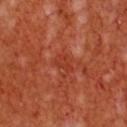Q: Was this lesion biopsied?
A: no biopsy performed (imaged during a skin exam)
Q: What kind of image is this?
A: 15 mm crop, total-body photography
Q: What is the lesion's diameter?
A: about 2.5 mm
Q: Who is the patient?
A: male, about 55 years old
Q: What did automated image analysis measure?
A: a shape eccentricity near 0.75 and a symmetry-axis asymmetry near 0.35; a mean CIELAB color near L≈42 a*≈36 b*≈37, a lesion–skin lightness drop of about 6, and a lesion-to-skin contrast of about 5.5 (normalized; higher = more distinct); a border-irregularity rating of about 3/10, a within-lesion color-variation index near 1.5/10, and a peripheral color-asymmetry measure near 0.5
Q: How was the tile lit?
A: cross-polarized illumination
Q: Where on the body is the lesion?
A: the front of the torso